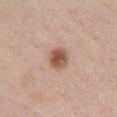Imaged with white-light lighting.
A female patient in their 40s.
The lesion is located on the chest.
Measured at roughly 3 mm in maximum diameter.
A 15 mm close-up tile from a total-body photography series done for melanoma screening.